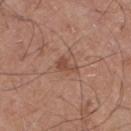{
  "biopsy_status": "not biopsied; imaged during a skin examination",
  "site": "leg",
  "image": {
    "source": "total-body photography crop",
    "field_of_view_mm": 15
  },
  "patient": {
    "sex": "male",
    "age_approx": 50
  },
  "lesion_size": {
    "long_diameter_mm_approx": 2.5
  },
  "lighting": "white-light"
}